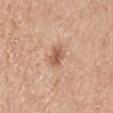Image and clinical context: A close-up tile cropped from a whole-body skin photograph, about 15 mm across. Automated image analysis of the tile measured a nevus-likeness score of about 75/100 and a detector confidence of about 100 out of 100 that the crop contains a lesion. From the right upper arm. This is a white-light tile. The subject is a male approximately 70 years of age. About 3 mm across.